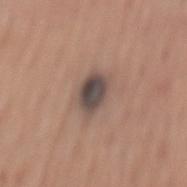workup = catalogued during a skin exam; not biopsied
acquisition = 15 mm crop, total-body photography
patient = female, aged 63 to 67
anatomic site = the back